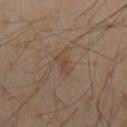This lesion was catalogued during total-body skin photography and was not selected for biopsy. Automated tile analysis of the lesion measured a lesion color around L≈44 a*≈15 b*≈27 in CIELAB, about 5 CIELAB-L* units darker than the surrounding skin, and a normalized border contrast of about 5. And it measured a nevus-likeness score of about 5/100. Measured at roughly 3 mm in maximum diameter. The tile uses cross-polarized illumination. A roughly 15 mm field-of-view crop from a total-body skin photograph. The lesion is on the mid back. The subject is a male aged approximately 55.